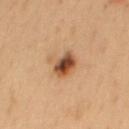{
  "biopsy_status": "not biopsied; imaged during a skin examination",
  "image": {
    "source": "total-body photography crop",
    "field_of_view_mm": 15
  },
  "lesion_size": {
    "long_diameter_mm_approx": 3.5
  },
  "site": "mid back",
  "lighting": "cross-polarized",
  "patient": {
    "sex": "male",
    "age_approx": 55
  }
}